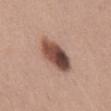biopsy_status: not biopsied; imaged during a skin examination
image:
  source: total-body photography crop
  field_of_view_mm: 15
site: mid back
patient:
  sex: male
  age_approx: 65